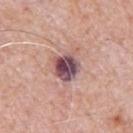notes: imaged on a skin check; not biopsied